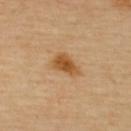Q: Was a biopsy performed?
A: no biopsy performed (imaged during a skin exam)
Q: Lesion size?
A: ≈4 mm
Q: Where on the body is the lesion?
A: the upper back
Q: What kind of image is this?
A: ~15 mm tile from a whole-body skin photo
Q: What did automated image analysis measure?
A: an area of roughly 7 mm² and two-axis asymmetry of about 0.3; a mean CIELAB color near L≈55 a*≈21 b*≈41, a lesion–skin lightness drop of about 11, and a lesion-to-skin contrast of about 8.5 (normalized; higher = more distinct); a border-irregularity index near 3/10, a within-lesion color-variation index near 4/10, and a peripheral color-asymmetry measure near 1
Q: Illumination type?
A: cross-polarized illumination
Q: Patient demographics?
A: female, aged 68–72